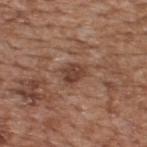Impression:
Recorded during total-body skin imaging; not selected for excision or biopsy.
Image and clinical context:
The lesion's longest dimension is about 3 mm. The patient is a male roughly 65 years of age. Located on the upper back. Imaged with white-light lighting. A region of skin cropped from a whole-body photographic capture, roughly 15 mm wide.